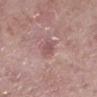| field | value |
|---|---|
| notes | no biopsy performed (imaged during a skin exam) |
| lighting | white-light illumination |
| image-analysis metrics | a mean CIELAB color near L≈53 a*≈21 b*≈20, a lesion–skin lightness drop of about 8, and a lesion-to-skin contrast of about 6 (normalized; higher = more distinct); a border-irregularity index near 3/10, a color-variation rating of about 1.5/10, and radial color variation of about 0.5; a classifier nevus-likeness of about 0/100 |
| size | about 3.5 mm |
| image | total-body-photography crop, ~15 mm field of view |
| subject | female, roughly 75 years of age |
| location | the left lower leg |